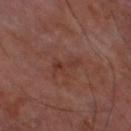<lesion>
<biopsy_status>not biopsied; imaged during a skin examination</biopsy_status>
<site>leg</site>
<image>
  <source>total-body photography crop</source>
  <field_of_view_mm>15</field_of_view_mm>
</image>
<patient>
  <sex>male</sex>
  <age_approx>70</age_approx>
</patient>
<lesion_size>
  <long_diameter_mm_approx>3.0</long_diameter_mm_approx>
</lesion_size>
<lighting>cross-polarized</lighting>
</lesion>